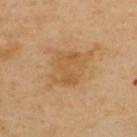Part of a total-body skin-imaging series; this lesion was reviewed on a skin check and was not flagged for biopsy.